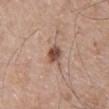notes: catalogued during a skin exam; not biopsied | anatomic site: the chest | tile lighting: white-light illumination | subject: male, approximately 55 years of age | imaging modality: ~15 mm crop, total-body skin-cancer survey.